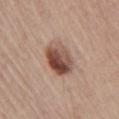notes: total-body-photography surveillance lesion; no biopsy
body site: the chest
imaging modality: ~15 mm tile from a whole-body skin photo
subject: male, aged 68 to 72
lesion diameter: about 4 mm
illumination: white-light illumination